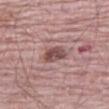Q: Was this lesion biopsied?
A: catalogued during a skin exam; not biopsied
Q: What are the patient's age and sex?
A: male, aged around 70
Q: What is the imaging modality?
A: ~15 mm crop, total-body skin-cancer survey
Q: What is the anatomic site?
A: the right thigh